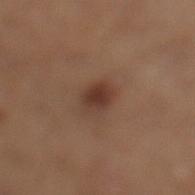Case summary:
- biopsy status · total-body-photography surveillance lesion; no biopsy
- lighting · white-light
- location · the left lower leg
- automated lesion analysis · an average lesion color of about L≈37 a*≈20 b*≈26 (CIELAB), a lesion–skin lightness drop of about 10, and a normalized lesion–skin contrast near 8.5
- image source · ~15 mm crop, total-body skin-cancer survey
- subject · male, aged 63 to 67